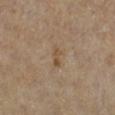<record>
<image>
  <source>total-body photography crop</source>
  <field_of_view_mm>15</field_of_view_mm>
</image>
<lighting>cross-polarized</lighting>
<site>right lower leg</site>
<automated_metrics>
  <area_mm2_approx>2.5</area_mm2_approx>
  <border_irregularity_0_10>4.0</border_irregularity_0_10>
  <color_variation_0_10>0.0</color_variation_0_10>
  <peripheral_color_asymmetry>0.0</peripheral_color_asymmetry>
</automated_metrics>
<patient>
  <sex>male</sex>
  <age_approx>85+</age_approx>
</patient>
<lesion_size>
  <long_diameter_mm_approx>2.5</long_diameter_mm_approx>
</lesion_size>
</record>